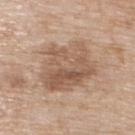<case>
  <biopsy_status>not biopsied; imaged during a skin examination</biopsy_status>
  <automated_metrics>
    <nevus_likeness_0_100>5</nevus_likeness_0_100>
  </automated_metrics>
  <lesion_size>
    <long_diameter_mm_approx>6.5</long_diameter_mm_approx>
  </lesion_size>
  <patient>
    <sex>female</sex>
    <age_approx>75</age_approx>
  </patient>
  <lighting>white-light</lighting>
  <image>
    <source>total-body photography crop</source>
    <field_of_view_mm>15</field_of_view_mm>
  </image>
  <site>upper back</site>
</case>